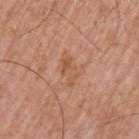Part of a total-body skin-imaging series; this lesion was reviewed on a skin check and was not flagged for biopsy. Cropped from a total-body skin-imaging series; the visible field is about 15 mm. Imaged with white-light lighting. Approximately 3.5 mm at its widest. A male subject, roughly 55 years of age. The lesion-visualizer software estimated an area of roughly 4.5 mm² and an outline eccentricity of about 0.85 (0 = round, 1 = elongated). The analysis additionally found a detector confidence of about 100 out of 100 that the crop contains a lesion. From the left upper arm.